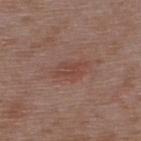The lesion is on the upper back. A roughly 15 mm field-of-view crop from a total-body skin photograph. The lesion-visualizer software estimated a footprint of about 7 mm² and an outline eccentricity of about 0.85 (0 = round, 1 = elongated). It also reported roughly 6 lightness units darker than nearby skin and a normalized lesion–skin contrast near 5.5. And it measured internal color variation of about 2 on a 0–10 scale and radial color variation of about 1. And it measured a classifier nevus-likeness of about 5/100 and a detector confidence of about 100 out of 100 that the crop contains a lesion. A male patient, in their 50s.